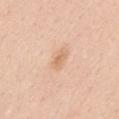Assessment:
Part of a total-body skin-imaging series; this lesion was reviewed on a skin check and was not flagged for biopsy.
Acquisition and patient details:
A roughly 15 mm field-of-view crop from a total-body skin photograph. A female subject, in their 40s. From the mid back.